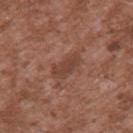notes: no biopsy performed (imaged during a skin exam); patient: male, aged 43–47; imaging modality: total-body-photography crop, ~15 mm field of view; lighting: white-light; site: the upper back.